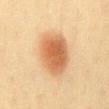| feature | finding |
|---|---|
| notes | total-body-photography surveillance lesion; no biopsy |
| diameter | ≈5.5 mm |
| image source | ~15 mm tile from a whole-body skin photo |
| subject | female, approximately 40 years of age |
| location | the front of the torso |
| automated lesion analysis | a footprint of about 18 mm², an outline eccentricity of about 0.65 (0 = round, 1 = elongated), and a shape-asymmetry score of about 0.15 (0 = symmetric); a mean CIELAB color near L≈54 a*≈21 b*≈36, about 13 CIELAB-L* units darker than the surrounding skin, and a normalized border contrast of about 9; a border-irregularity rating of about 1.5/10, internal color variation of about 4 on a 0–10 scale, and peripheral color asymmetry of about 1 |
| illumination | cross-polarized |